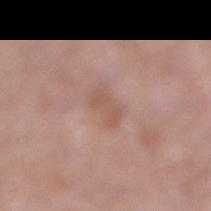The lesion was photographed on a routine skin check and not biopsied; there is no pathology result.
The lesion is located on the right lower leg.
A 15 mm crop from a total-body photograph taken for skin-cancer surveillance.
A male patient, aged approximately 75.